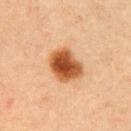Part of a total-body skin-imaging series; this lesion was reviewed on a skin check and was not flagged for biopsy.
Located on the right upper arm.
The total-body-photography lesion software estimated about 17 CIELAB-L* units darker than the surrounding skin. The analysis additionally found a border-irregularity rating of about 1.5/10 and internal color variation of about 5.5 on a 0–10 scale.
Longest diameter approximately 4.5 mm.
Captured under cross-polarized illumination.
A 15 mm close-up extracted from a 3D total-body photography capture.
A female subject, about 45 years old.